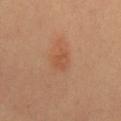The subject is a female approximately 50 years of age. Automated image analysis of the tile measured a lesion–skin lightness drop of about 6 and a normalized lesion–skin contrast near 5. And it measured a border-irregularity index near 3/10, internal color variation of about 2 on a 0–10 scale, and radial color variation of about 1. The analysis additionally found a classifier nevus-likeness of about 30/100 and lesion-presence confidence of about 100/100. Cropped from a total-body skin-imaging series; the visible field is about 15 mm. Longest diameter approximately 3 mm. This is a cross-polarized tile.